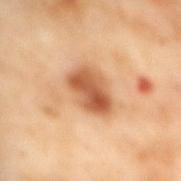Case summary:
• follow-up: imaged on a skin check; not biopsied
• patient: female, about 55 years old
• site: the mid back
• imaging modality: ~15 mm tile from a whole-body skin photo
• lesion size: ~4.5 mm (longest diameter)
• lighting: cross-polarized illumination
• automated lesion analysis: a border-irregularity rating of about 2/10